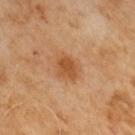Q: Was this lesion biopsied?
A: catalogued during a skin exam; not biopsied
Q: What is the lesion's diameter?
A: about 3.5 mm
Q: What is the imaging modality?
A: ~15 mm tile from a whole-body skin photo
Q: Patient demographics?
A: male, in their mid- to late 60s